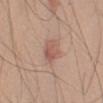Recorded during total-body skin imaging; not selected for excision or biopsy. The recorded lesion diameter is about 2.5 mm. A male patient, in their mid-40s. Located on the left upper arm. Automated image analysis of the tile measured an area of roughly 5.5 mm² and a shape eccentricity near 0.35. It also reported a border-irregularity rating of about 2/10, a color-variation rating of about 3.5/10, and a peripheral color-asymmetry measure near 1.5. And it measured a nevus-likeness score of about 20/100. Cropped from a whole-body photographic skin survey; the tile spans about 15 mm. Imaged with white-light lighting.